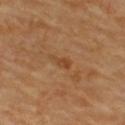Captured during whole-body skin photography for melanoma surveillance; the lesion was not biopsied.
Cropped from a whole-body photographic skin survey; the tile spans about 15 mm.
About 2.5 mm across.
Located on the upper back.
A female subject, aged 58–62.